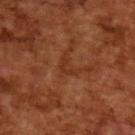<lesion>
<biopsy_status>not biopsied; imaged during a skin examination</biopsy_status>
<patient>
  <sex>male</sex>
  <age_approx>65</age_approx>
</patient>
<image>
  <source>total-body photography crop</source>
  <field_of_view_mm>15</field_of_view_mm>
</image>
</lesion>